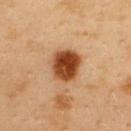Findings:
• workup · total-body-photography surveillance lesion; no biopsy
• body site · the upper back
• acquisition · ~15 mm tile from a whole-body skin photo
• patient · male, aged approximately 40